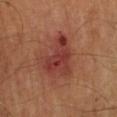Captured during whole-body skin photography for melanoma surveillance; the lesion was not biopsied.
Located on the left lower leg.
A male subject, approximately 65 years of age.
Measured at roughly 5 mm in maximum diameter.
A 15 mm crop from a total-body photograph taken for skin-cancer surveillance.